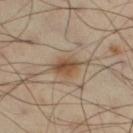Clinical summary:
Located on the right thigh. Automated tile analysis of the lesion measured an eccentricity of roughly 0.75 and a shape-asymmetry score of about 0.15 (0 = symmetric). It also reported a lesion–skin lightness drop of about 11 and a normalized lesion–skin contrast near 9. The recorded lesion diameter is about 3.5 mm. A 15 mm close-up extracted from a 3D total-body photography capture. A male subject about 55 years old. The tile uses cross-polarized illumination.